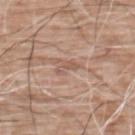No biopsy was performed on this lesion — it was imaged during a full skin examination and was not determined to be concerning. A lesion tile, about 15 mm wide, cut from a 3D total-body photograph. The lesion's longest dimension is about 3 mm. The lesion is located on the upper back. The tile uses white-light illumination. The patient is a male roughly 60 years of age.